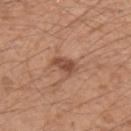The lesion was photographed on a routine skin check and not biopsied; there is no pathology result. The patient is a male about 30 years old. On the left upper arm. Imaged with white-light lighting. Automated image analysis of the tile measured an outline eccentricity of about 0.75 (0 = round, 1 = elongated) and two-axis asymmetry of about 0.35. Approximately 3 mm at its widest. Cropped from a whole-body photographic skin survey; the tile spans about 15 mm.